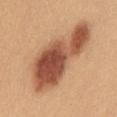This lesion was catalogued during total-body skin photography and was not selected for biopsy.
A lesion tile, about 15 mm wide, cut from a 3D total-body photograph.
The lesion is on the mid back.
This is a white-light tile.
The patient is a female aged 23 to 27.